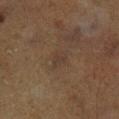Acquisition and patient details:
The tile uses cross-polarized illumination. A male subject aged around 65. Cropped from a whole-body photographic skin survey; the tile spans about 15 mm. The total-body-photography lesion software estimated an automated nevus-likeness rating near 0 out of 100 and a lesion-detection confidence of about 90/100. From the right lower leg. Longest diameter approximately 2.5 mm.